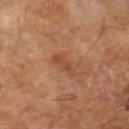Imaged during a routine full-body skin examination; the lesion was not biopsied and no histopathology is available. Longest diameter approximately 3.5 mm. This is a cross-polarized tile. The subject is a male about 65 years old. The total-body-photography lesion software estimated internal color variation of about 2 on a 0–10 scale and radial color variation of about 0.5. The analysis additionally found a classifier nevus-likeness of about 0/100 and lesion-presence confidence of about 100/100. A 15 mm close-up tile from a total-body photography series done for melanoma screening.